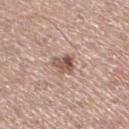This lesion was catalogued during total-body skin photography and was not selected for biopsy. Cropped from a whole-body photographic skin survey; the tile spans about 15 mm. The patient is a male roughly 65 years of age. Located on the left lower leg.